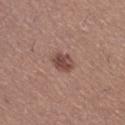Case summary:
• body site: the right thigh
• automated metrics: a footprint of about 5.5 mm² and a shape eccentricity near 0.45; a normalized lesion–skin contrast near 8; a detector confidence of about 100 out of 100 that the crop contains a lesion
• lighting: white-light
• subject: female, aged approximately 25
• image source: ~15 mm crop, total-body skin-cancer survey
• lesion diameter: ≈2.5 mm